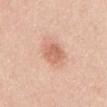follow-up: total-body-photography surveillance lesion; no biopsy
image source: ~15 mm tile from a whole-body skin photo
subject: male, approximately 50 years of age
body site: the abdomen